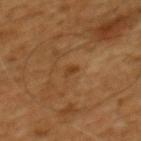Imaged during a routine full-body skin examination; the lesion was not biopsied and no histopathology is available.
The subject is a male aged around 45.
The lesion is located on the mid back.
A 15 mm close-up extracted from a 3D total-body photography capture.